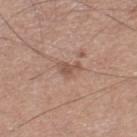The lesion was photographed on a routine skin check and not biopsied; there is no pathology result.
Automated tile analysis of the lesion measured a lesion area of about 3.5 mm², an outline eccentricity of about 0.7 (0 = round, 1 = elongated), and a shape-asymmetry score of about 0.5 (0 = symmetric). It also reported border irregularity of about 5 on a 0–10 scale, internal color variation of about 1 on a 0–10 scale, and radial color variation of about 0.5.
A male patient, in their 50s.
A close-up tile cropped from a whole-body skin photograph, about 15 mm across.
Measured at roughly 2.5 mm in maximum diameter.
The lesion is located on the right lower leg.